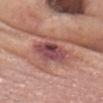This lesion was catalogued during total-body skin photography and was not selected for biopsy. A male subject, in their mid-60s. A close-up tile cropped from a whole-body skin photograph, about 15 mm across. The total-body-photography lesion software estimated an area of roughly 15 mm², an outline eccentricity of about 0.8 (0 = round, 1 = elongated), and two-axis asymmetry of about 0.35. The analysis additionally found border irregularity of about 4 on a 0–10 scale and a color-variation rating of about 8/10. The lesion is located on the head or neck.